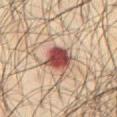{
  "biopsy_status": "not biopsied; imaged during a skin examination",
  "automated_metrics": {
    "area_mm2_approx": 9.5,
    "eccentricity": 0.3,
    "cielab_L": 40,
    "cielab_a": 22,
    "cielab_b": 21,
    "vs_skin_darker_L": 16.0,
    "vs_skin_contrast_norm": 12.5,
    "border_irregularity_0_10": 2.0,
    "color_variation_0_10": 5.5,
    "peripheral_color_asymmetry": 2.0
  },
  "patient": {
    "sex": "male",
    "age_approx": 65
  },
  "site": "abdomen",
  "image": {
    "source": "total-body photography crop",
    "field_of_view_mm": 15
  },
  "lighting": "cross-polarized"
}